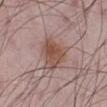Case summary:
- biopsy status — imaged on a skin check; not biopsied
- image source — total-body-photography crop, ~15 mm field of view
- subject — male, in their 50s
- lesion size — ≈4.5 mm
- location — the left lower leg
- automated lesion analysis — an area of roughly 12 mm², an eccentricity of roughly 0.45, and a symmetry-axis asymmetry near 0.35; lesion-presence confidence of about 100/100